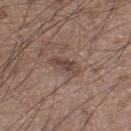Notes:
– body site — the right lower leg
– automated lesion analysis — an outline eccentricity of about 0.9 (0 = round, 1 = elongated) and two-axis asymmetry of about 0.35; a mean CIELAB color near L≈44 a*≈17 b*≈22 and a lesion–skin lightness drop of about 9; border irregularity of about 4.5 on a 0–10 scale and peripheral color asymmetry of about 0.5
– image source — total-body-photography crop, ~15 mm field of view
– tile lighting — white-light illumination
– subject — male, roughly 20 years of age
– lesion diameter — ≈3.5 mm